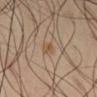follow-up: catalogued during a skin exam; not biopsied
acquisition: total-body-photography crop, ~15 mm field of view
lesion diameter: about 2.5 mm
body site: the leg
subject: male, approximately 60 years of age